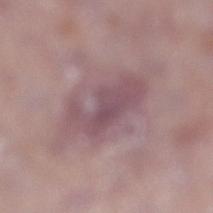Imaged during a routine full-body skin examination; the lesion was not biopsied and no histopathology is available.
The lesion is located on the leg.
The patient is a male aged approximately 75.
Automated image analysis of the tile measured border irregularity of about 5.5 on a 0–10 scale, internal color variation of about 3.5 on a 0–10 scale, and peripheral color asymmetry of about 1. The analysis additionally found a nevus-likeness score of about 0/100.
A lesion tile, about 15 mm wide, cut from a 3D total-body photograph.
Longest diameter approximately 7 mm.